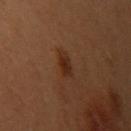Imaged during a routine full-body skin examination; the lesion was not biopsied and no histopathology is available. On the left upper arm. Imaged with cross-polarized lighting. A 15 mm crop from a total-body photograph taken for skin-cancer surveillance. The subject is a female about 50 years old. The total-body-photography lesion software estimated border irregularity of about 2.5 on a 0–10 scale, a within-lesion color-variation index near 2/10, and radial color variation of about 0.5. Longest diameter approximately 3 mm.